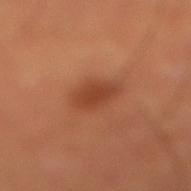follow-up: total-body-photography surveillance lesion; no biopsy | automated lesion analysis: a mean CIELAB color near L≈43 a*≈28 b*≈35 and a lesion-to-skin contrast of about 7 (normalized; higher = more distinct); a border-irregularity index near 2/10, a color-variation rating of about 2/10, and peripheral color asymmetry of about 0.5; a classifier nevus-likeness of about 80/100 and a lesion-detection confidence of about 100/100 | location: the right lower leg | imaging modality: 15 mm crop, total-body photography | subject: male, approximately 50 years of age | lesion diameter: about 4 mm.